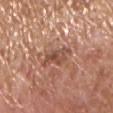Q: Was a biopsy performed?
A: total-body-photography surveillance lesion; no biopsy
Q: Lesion size?
A: ≈4 mm
Q: How was this image acquired?
A: ~15 mm tile from a whole-body skin photo
Q: Illumination type?
A: white-light
Q: Who is the patient?
A: male, aged 63 to 67
Q: What did automated image analysis measure?
A: a lesion color around L≈50 a*≈23 b*≈29 in CIELAB, about 10 CIELAB-L* units darker than the surrounding skin, and a normalized lesion–skin contrast near 7; a border-irregularity index near 4.5/10 and a color-variation rating of about 5.5/10
Q: Lesion location?
A: the chest